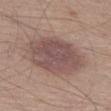follow-up: no biopsy performed (imaged during a skin exam) | lesion diameter: ≈7.5 mm | image source: ~15 mm tile from a whole-body skin photo | subject: male, aged 58 to 62 | location: the left thigh | image-analysis metrics: a lesion color around L≈50 a*≈17 b*≈20 in CIELAB, a lesion–skin lightness drop of about 10, and a lesion-to-skin contrast of about 7.5 (normalized; higher = more distinct); a border-irregularity rating of about 1.5/10, a within-lesion color-variation index near 3.5/10, and a peripheral color-asymmetry measure near 1; an automated nevus-likeness rating near 35 out of 100 and a detector confidence of about 100 out of 100 that the crop contains a lesion.